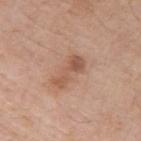notes — no biopsy performed (imaged during a skin exam) | subject — male, approximately 75 years of age | image source — 15 mm crop, total-body photography | body site — the right upper arm.